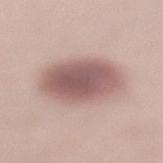No biopsy was performed on this lesion — it was imaged during a full skin examination and was not determined to be concerning. A 15 mm crop from a total-body photograph taken for skin-cancer surveillance. The patient is a female aged approximately 25. Located on the left lower leg. The total-body-photography lesion software estimated a classifier nevus-likeness of about 65/100 and lesion-presence confidence of about 100/100. The lesion's longest dimension is about 6 mm. The tile uses white-light illumination.